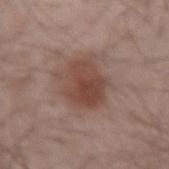{"biopsy_status": "not biopsied; imaged during a skin examination", "lesion_size": {"long_diameter_mm_approx": 5.0}, "image": {"source": "total-body photography crop", "field_of_view_mm": 15}, "patient": {"sex": "male", "age_approx": 55}, "automated_metrics": {"area_mm2_approx": 17.0, "eccentricity": 0.5, "shape_asymmetry": 0.25, "cielab_L": 45, "cielab_a": 19, "cielab_b": 24, "vs_skin_darker_L": 10.0, "vs_skin_contrast_norm": 8.0, "border_irregularity_0_10": 3.0, "color_variation_0_10": 4.5, "peripheral_color_asymmetry": 1.5, "nevus_likeness_0_100": 75, "lesion_detection_confidence_0_100": 100}, "site": "left forearm"}